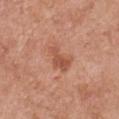Impression:
No biopsy was performed on this lesion — it was imaged during a full skin examination and was not determined to be concerning.
Background:
A 15 mm close-up tile from a total-body photography series done for melanoma screening. The subject is a female aged around 65. The lesion is located on the chest.